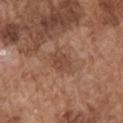A male subject in their mid- to late 70s. The lesion is on the right upper arm. A 15 mm close-up tile from a total-body photography series done for melanoma screening. An algorithmic analysis of the crop reported a footprint of about 5.5 mm², a shape eccentricity near 0.7, and two-axis asymmetry of about 0.25. It also reported a border-irregularity index near 2.5/10, a color-variation rating of about 2/10, and radial color variation of about 0.5. The software also gave an automated nevus-likeness rating near 0 out of 100.